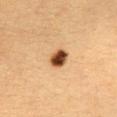Case summary:
• lesion diameter — about 3 mm
• body site — the upper back
• lighting — cross-polarized
• imaging modality — 15 mm crop, total-body photography
• patient — female, approximately 30 years of age